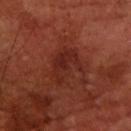biopsy_status: not biopsied; imaged during a skin examination
image:
  source: total-body photography crop
  field_of_view_mm: 15
lesion_size:
  long_diameter_mm_approx: 4.5
patient:
  sex: male
  age_approx: 70
site: front of the torso
automated_metrics:
  cielab_L: 26
  cielab_a: 26
  cielab_b: 26
  vs_skin_darker_L: 6.0
  border_irregularity_0_10: 5.0
  color_variation_0_10: 3.0
  peripheral_color_asymmetry: 1.0